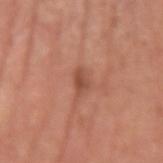Case summary:
• biopsy status — catalogued during a skin exam; not biopsied
• size — ~2.5 mm (longest diameter)
• patient — male, about 55 years old
• location — the left upper arm
• image source — 15 mm crop, total-body photography
• illumination — white-light illumination